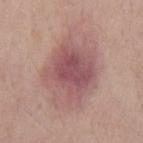Part of a total-body skin-imaging series; this lesion was reviewed on a skin check and was not flagged for biopsy. The subject is a male aged around 50. The tile uses white-light illumination. Approximately 9 mm at its widest. Automated image analysis of the tile measured a border-irregularity index near 2.5/10, a within-lesion color-variation index near 6/10, and peripheral color asymmetry of about 1. It also reported a lesion-detection confidence of about 100/100. Located on the mid back. A 15 mm crop from a total-body photograph taken for skin-cancer surveillance.